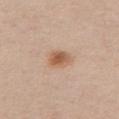Q: What is the lesion's diameter?
A: about 3 mm
Q: What lighting was used for the tile?
A: white-light illumination
Q: Where on the body is the lesion?
A: the chest
Q: Automated lesion metrics?
A: a lesion color around L≈58 a*≈20 b*≈32 in CIELAB, roughly 11 lightness units darker than nearby skin, and a normalized border contrast of about 8
Q: What are the patient's age and sex?
A: female, about 55 years old
Q: How was this image acquired?
A: ~15 mm crop, total-body skin-cancer survey